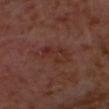follow-up: total-body-photography surveillance lesion; no biopsy | body site: the head or neck | tile lighting: cross-polarized illumination | patient: male, aged approximately 55 | image source: ~15 mm tile from a whole-body skin photo.